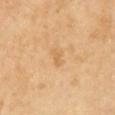Recorded during total-body skin imaging; not selected for excision or biopsy. A male subject, aged 63 to 67. Approximately 2.5 mm at its widest. A close-up tile cropped from a whole-body skin photograph, about 15 mm across. Captured under cross-polarized illumination.